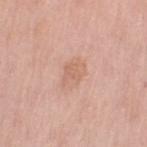{"biopsy_status": "not biopsied; imaged during a skin examination", "image": {"source": "total-body photography crop", "field_of_view_mm": 15}, "patient": {"sex": "female", "age_approx": 60}, "lesion_size": {"long_diameter_mm_approx": 3.0}, "lighting": "white-light", "site": "left upper arm"}